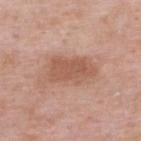follow-up: imaged on a skin check; not biopsied | site: the upper back | subject: male, in their mid- to late 50s | acquisition: 15 mm crop, total-body photography.